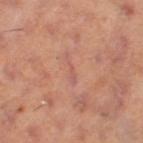Part of a total-body skin-imaging series; this lesion was reviewed on a skin check and was not flagged for biopsy. Measured at roughly 3.5 mm in maximum diameter. A 15 mm close-up tile from a total-body photography series done for melanoma screening. The subject is a female approximately 40 years of age. On the leg. The total-body-photography lesion software estimated a footprint of about 2.5 mm² and a symmetry-axis asymmetry near 0.45. The analysis additionally found a mean CIELAB color near L≈56 a*≈25 b*≈26, roughly 5 lightness units darker than nearby skin, and a lesion-to-skin contrast of about 4.5 (normalized; higher = more distinct). The software also gave a border-irregularity index near 6/10, internal color variation of about 0 on a 0–10 scale, and peripheral color asymmetry of about 0. Captured under cross-polarized illumination.